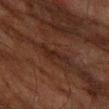lighting=cross-polarized illumination; anatomic site=the arm; diameter=about 3.5 mm; patient=male, aged around 65; image=15 mm crop, total-body photography.